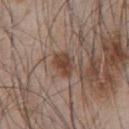biopsy status: no biopsy performed (imaged during a skin exam).